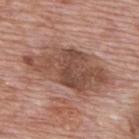workup = imaged on a skin check; not biopsied
tile lighting = white-light illumination
body site = the upper back
image = ~15 mm crop, total-body skin-cancer survey
diameter = ~10 mm (longest diameter)
automated lesion analysis = a mean CIELAB color near L≈49 a*≈20 b*≈27 and roughly 12 lightness units darker than nearby skin; a classifier nevus-likeness of about 0/100
patient = male, roughly 70 years of age